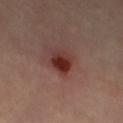<record>
<biopsy_status>not biopsied; imaged during a skin examination</biopsy_status>
<lighting>cross-polarized</lighting>
<patient>
  <sex>female</sex>
  <age_approx>65</age_approx>
</patient>
<site>left thigh</site>
<image>
  <source>total-body photography crop</source>
  <field_of_view_mm>15</field_of_view_mm>
</image>
<automated_metrics>
  <area_mm2_approx>7.0</area_mm2_approx>
  <eccentricity>0.55</eccentricity>
  <shape_asymmetry>0.2</shape_asymmetry>
  <border_irregularity_0_10>1.5</border_irregularity_0_10>
</automated_metrics>
</record>